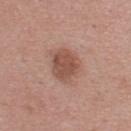Q: Is there a histopathology result?
A: total-body-photography surveillance lesion; no biopsy